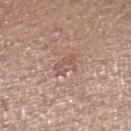biopsy status: total-body-photography surveillance lesion; no biopsy
body site: the right lower leg
patient: male, in their mid-60s
illumination: white-light
image source: ~15 mm tile from a whole-body skin photo
lesion size: about 3 mm